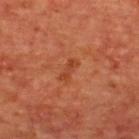Acquisition and patient details: From the upper back. Measured at roughly 3 mm in maximum diameter. A roughly 15 mm field-of-view crop from a total-body skin photograph. A male subject, in their mid- to late 60s.